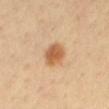No biopsy was performed on this lesion — it was imaged during a full skin examination and was not determined to be concerning.